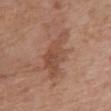Assessment:
Part of a total-body skin-imaging series; this lesion was reviewed on a skin check and was not flagged for biopsy.
Acquisition and patient details:
About 6.5 mm across. From the chest. Automated tile analysis of the lesion measured an average lesion color of about L≈49 a*≈21 b*≈29 (CIELAB), roughly 8 lightness units darker than nearby skin, and a lesion-to-skin contrast of about 6 (normalized; higher = more distinct). The analysis additionally found a border-irregularity rating of about 5.5/10, a color-variation rating of about 4/10, and radial color variation of about 1.5. The analysis additionally found a detector confidence of about 100 out of 100 that the crop contains a lesion. This image is a 15 mm lesion crop taken from a total-body photograph. The patient is a female roughly 75 years of age.